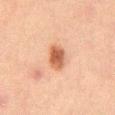Impression:
No biopsy was performed on this lesion — it was imaged during a full skin examination and was not determined to be concerning.
Context:
Cropped from a whole-body photographic skin survey; the tile spans about 15 mm. A male patient, aged 63 to 67. The total-body-photography lesion software estimated a lesion area of about 5.5 mm², an eccentricity of roughly 0.75, and a shape-asymmetry score of about 0.15 (0 = symmetric). The software also gave a lesion color around L≈47 a*≈22 b*≈31 in CIELAB, about 12 CIELAB-L* units darker than the surrounding skin, and a normalized lesion–skin contrast near 9.5. The software also gave a border-irregularity index near 1.5/10, internal color variation of about 3 on a 0–10 scale, and radial color variation of about 1. The analysis additionally found an automated nevus-likeness rating near 100 out of 100 and a detector confidence of about 100 out of 100 that the crop contains a lesion. Imaged with cross-polarized lighting. On the mid back.